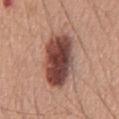Impression:
This lesion was catalogued during total-body skin photography and was not selected for biopsy.
Context:
Captured under white-light illumination. This image is a 15 mm lesion crop taken from a total-body photograph. The lesion is located on the abdomen. The total-body-photography lesion software estimated a lesion area of about 24 mm², an outline eccentricity of about 0.9 (0 = round, 1 = elongated), and a shape-asymmetry score of about 0.15 (0 = symmetric). The software also gave a lesion color around L≈45 a*≈23 b*≈24 in CIELAB and a lesion-to-skin contrast of about 13 (normalized; higher = more distinct). And it measured internal color variation of about 8 on a 0–10 scale and a peripheral color-asymmetry measure near 2.5. The software also gave a nevus-likeness score of about 60/100 and lesion-presence confidence of about 100/100. A male subject about 60 years old. About 8 mm across.